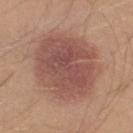The lesion was photographed on a routine skin check and not biopsied; there is no pathology result.
The subject is a male aged around 30.
The lesion is on the left lower leg.
Approximately 8 mm at its widest.
A 15 mm close-up tile from a total-body photography series done for melanoma screening.